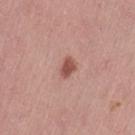From the left thigh.
The subject is a female aged 48 to 52.
Measured at roughly 2.5 mm in maximum diameter.
A region of skin cropped from a whole-body photographic capture, roughly 15 mm wide.
The total-body-photography lesion software estimated an average lesion color of about L≈52 a*≈25 b*≈27 (CIELAB), a lesion–skin lightness drop of about 12, and a normalized lesion–skin contrast near 8.5.
Captured under white-light illumination.